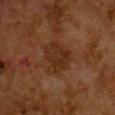Q: Was this lesion biopsied?
A: total-body-photography surveillance lesion; no biopsy
Q: Where on the body is the lesion?
A: the chest
Q: Illumination type?
A: cross-polarized
Q: What kind of image is this?
A: 15 mm crop, total-body photography
Q: What is the lesion's diameter?
A: about 4 mm
Q: Patient demographics?
A: female, aged approximately 50
Q: What did automated image analysis measure?
A: border irregularity of about 2.5 on a 0–10 scale, a within-lesion color-variation index near 2.5/10, and peripheral color asymmetry of about 1; a nevus-likeness score of about 0/100 and a lesion-detection confidence of about 100/100